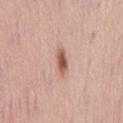Q: Was a biopsy performed?
A: catalogued during a skin exam; not biopsied
Q: What are the patient's age and sex?
A: female, aged 48–52
Q: How large is the lesion?
A: about 3.5 mm
Q: What kind of image is this?
A: ~15 mm tile from a whole-body skin photo
Q: Where on the body is the lesion?
A: the abdomen
Q: What lighting was used for the tile?
A: white-light